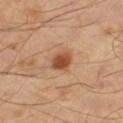This lesion was catalogued during total-body skin photography and was not selected for biopsy. The lesion's longest dimension is about 3 mm. The lesion is on the leg. A region of skin cropped from a whole-body photographic capture, roughly 15 mm wide. Imaged with cross-polarized lighting.